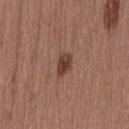Impression: No biopsy was performed on this lesion — it was imaged during a full skin examination and was not determined to be concerning. Clinical summary: The total-body-photography lesion software estimated a mean CIELAB color near L≈40 a*≈21 b*≈24, about 12 CIELAB-L* units darker than the surrounding skin, and a normalized lesion–skin contrast near 9.5. Approximately 3 mm at its widest. From the lower back. The subject is a female aged 38–42. This image is a 15 mm lesion crop taken from a total-body photograph.